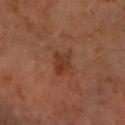Clinical impression: Recorded during total-body skin imaging; not selected for excision or biopsy. Background: A roughly 15 mm field-of-view crop from a total-body skin photograph. A female patient aged around 60. On the left forearm. This is a cross-polarized tile. About 3 mm across.